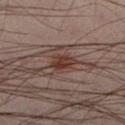This lesion was catalogued during total-body skin photography and was not selected for biopsy. On the left lower leg. An algorithmic analysis of the crop reported a lesion area of about 5.5 mm², an outline eccentricity of about 0.75 (0 = round, 1 = elongated), and a symmetry-axis asymmetry near 0.25. And it measured a lesion color around L≈28 a*≈16 b*≈19 in CIELAB, a lesion–skin lightness drop of about 8, and a normalized lesion–skin contrast near 8.5. The analysis additionally found border irregularity of about 2.5 on a 0–10 scale, a color-variation rating of about 3/10, and a peripheral color-asymmetry measure near 1. The software also gave a nevus-likeness score of about 95/100. The patient is a male in their 40s. This is a cross-polarized tile. Cropped from a whole-body photographic skin survey; the tile spans about 15 mm.